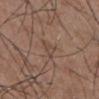Q: Lesion size?
A: ~3 mm (longest diameter)
Q: How was this image acquired?
A: 15 mm crop, total-body photography
Q: What did automated image analysis measure?
A: an area of roughly 3.5 mm² and an outline eccentricity of about 0.85 (0 = round, 1 = elongated); a lesion color around L≈45 a*≈16 b*≈24 in CIELAB and roughly 6 lightness units darker than nearby skin
Q: Who is the patient?
A: male, aged 53–57
Q: How was the tile lit?
A: white-light illumination
Q: Where on the body is the lesion?
A: the abdomen